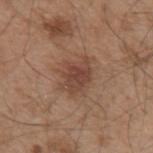Q: Was this lesion biopsied?
A: total-body-photography surveillance lesion; no biopsy
Q: Lesion location?
A: the left upper arm
Q: What lighting was used for the tile?
A: white-light illumination
Q: Patient demographics?
A: male, aged approximately 55
Q: What is the imaging modality?
A: ~15 mm crop, total-body skin-cancer survey
Q: Automated lesion metrics?
A: roughly 9 lightness units darker than nearby skin and a lesion-to-skin contrast of about 7.5 (normalized; higher = more distinct)
Q: How large is the lesion?
A: about 4 mm